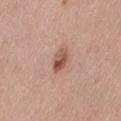notes — total-body-photography surveillance lesion; no biopsy | imaging modality — ~15 mm crop, total-body skin-cancer survey | lighting — white-light illumination | TBP lesion metrics — internal color variation of about 6.5 on a 0–10 scale and radial color variation of about 2.5 | patient — female, in their 40s | size — ≈3.5 mm | body site — the left thigh.